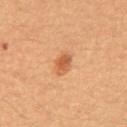Clinical impression: Imaged during a routine full-body skin examination; the lesion was not biopsied and no histopathology is available. Context: A close-up tile cropped from a whole-body skin photograph, about 15 mm across. Located on the abdomen. Longest diameter approximately 2.5 mm. A male subject, approximately 50 years of age.